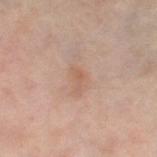<lesion>
<biopsy_status>not biopsied; imaged during a skin examination</biopsy_status>
<automated_metrics>
  <vs_skin_darker_L>6.0</vs_skin_darker_L>
  <vs_skin_contrast_norm>5.0</vs_skin_contrast_norm>
</automated_metrics>
<site>left thigh</site>
<image>
  <source>total-body photography crop</source>
  <field_of_view_mm>15</field_of_view_mm>
</image>
<lighting>cross-polarized</lighting>
<patient>
  <sex>female</sex>
  <age_approx>50</age_approx>
</patient>
</lesion>